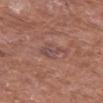Assessment:
Imaged during a routine full-body skin examination; the lesion was not biopsied and no histopathology is available.
Clinical summary:
From the left thigh. Measured at roughly 3 mm in maximum diameter. A 15 mm crop from a total-body photograph taken for skin-cancer surveillance. Automated image analysis of the tile measured an area of roughly 6 mm², an outline eccentricity of about 0.6 (0 = round, 1 = elongated), and a shape-asymmetry score of about 0.25 (0 = symmetric). And it measured border irregularity of about 3.5 on a 0–10 scale and a within-lesion color-variation index near 3.5/10. A male subject in their mid-60s. This is a white-light tile.